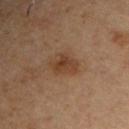{"biopsy_status": "not biopsied; imaged during a skin examination"}